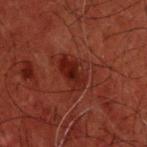– biopsy status: total-body-photography surveillance lesion; no biopsy
– anatomic site: the head or neck
– imaging modality: total-body-photography crop, ~15 mm field of view
– patient: male, aged 58 to 62
– TBP lesion metrics: an area of roughly 8 mm² and an eccentricity of roughly 0.85; border irregularity of about 2.5 on a 0–10 scale, a color-variation rating of about 4/10, and a peripheral color-asymmetry measure near 1.5; a lesion-detection confidence of about 100/100
– lesion diameter: ≈4.5 mm